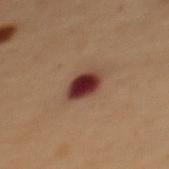No biopsy was performed on this lesion — it was imaged during a full skin examination and was not determined to be concerning. A female subject aged approximately 50. Measured at roughly 3 mm in maximum diameter. Captured under cross-polarized illumination. A roughly 15 mm field-of-view crop from a total-body skin photograph. The lesion is on the upper back. The total-body-photography lesion software estimated a border-irregularity index near 2/10, a within-lesion color-variation index near 5/10, and a peripheral color-asymmetry measure near 1.